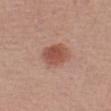No biopsy was performed on this lesion — it was imaged during a full skin examination and was not determined to be concerning. A lesion tile, about 15 mm wide, cut from a 3D total-body photograph. The lesion is on the left forearm. Imaged with white-light lighting. Longest diameter approximately 3.5 mm. A male subject, roughly 60 years of age.